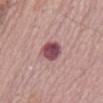{
  "biopsy_status": "not biopsied; imaged during a skin examination",
  "lighting": "white-light",
  "site": "front of the torso",
  "lesion_size": {
    "long_diameter_mm_approx": 3.0
  },
  "patient": {
    "sex": "male",
    "age_approx": 70
  },
  "image": {
    "source": "total-body photography crop",
    "field_of_view_mm": 15
  }
}